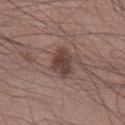The lesion was tiled from a total-body skin photograph and was not biopsied. The patient is a male aged 38 to 42. From the right lower leg. The lesion-visualizer software estimated a footprint of about 6.5 mm², a shape eccentricity near 0.75, and a symmetry-axis asymmetry near 0.2. It also reported a mean CIELAB color near L≈40 a*≈18 b*≈21 and a lesion-to-skin contrast of about 9.5 (normalized; higher = more distinct). And it measured a border-irregularity index near 2/10, a color-variation rating of about 2.5/10, and radial color variation of about 1. Approximately 3.5 mm at its widest. A region of skin cropped from a whole-body photographic capture, roughly 15 mm wide. This is a white-light tile.